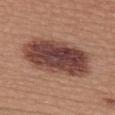The lesion was tiled from a total-body skin photograph and was not biopsied. The lesion is located on the upper back. A region of skin cropped from a whole-body photographic capture, roughly 15 mm wide. A female subject, about 20 years old.